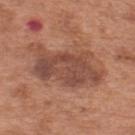The lesion was photographed on a routine skin check and not biopsied; there is no pathology result.
A male subject, aged 63–67.
A 15 mm close-up tile from a total-body photography series done for melanoma screening.
The tile uses white-light illumination.
Approximately 9 mm at its widest.
The total-body-photography lesion software estimated about 10 CIELAB-L* units darker than the surrounding skin and a lesion-to-skin contrast of about 8 (normalized; higher = more distinct). And it measured a border-irregularity rating of about 5.5/10, internal color variation of about 4.5 on a 0–10 scale, and radial color variation of about 1.5. The software also gave an automated nevus-likeness rating near 0 out of 100.
On the upper back.